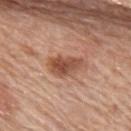Clinical impression:
This lesion was catalogued during total-body skin photography and was not selected for biopsy.
Background:
The patient is a female aged approximately 65. Located on the upper back. The lesion's longest dimension is about 4.5 mm. This is a white-light tile. Automated tile analysis of the lesion measured a lesion area of about 9 mm² and a shape eccentricity near 0.85. And it measured a color-variation rating of about 5/10 and radial color variation of about 1.5. It also reported an automated nevus-likeness rating near 90 out of 100 and a lesion-detection confidence of about 100/100. A 15 mm crop from a total-body photograph taken for skin-cancer surveillance.